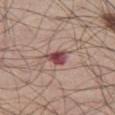The lesion was tiled from a total-body skin photograph and was not biopsied. From the left thigh. Imaged with white-light lighting. Longest diameter approximately 3 mm. A male patient aged 53–57. A lesion tile, about 15 mm wide, cut from a 3D total-body photograph.